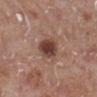This lesion was catalogued during total-body skin photography and was not selected for biopsy. The lesion's longest dimension is about 3.5 mm. The tile uses white-light illumination. A female subject, aged around 75. This image is a 15 mm lesion crop taken from a total-body photograph. From the left lower leg.